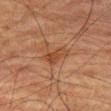Q: Is there a histopathology result?
A: no biopsy performed (imaged during a skin exam)
Q: What is the lesion's diameter?
A: ~3.5 mm (longest diameter)
Q: What did automated image analysis measure?
A: an average lesion color of about L≈35 a*≈20 b*≈28 (CIELAB), about 7 CIELAB-L* units darker than the surrounding skin, and a normalized lesion–skin contrast near 6.5; a nevus-likeness score of about 10/100 and a detector confidence of about 100 out of 100 that the crop contains a lesion
Q: What are the patient's age and sex?
A: male, approximately 80 years of age
Q: Illumination type?
A: cross-polarized illumination
Q: What kind of image is this?
A: 15 mm crop, total-body photography
Q: What is the anatomic site?
A: the left thigh